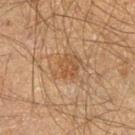Findings:
* notes: imaged on a skin check; not biopsied